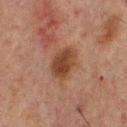Q: Was this lesion biopsied?
A: total-body-photography surveillance lesion; no biopsy
Q: Lesion location?
A: the upper back
Q: How was this image acquired?
A: total-body-photography crop, ~15 mm field of view
Q: Automated lesion metrics?
A: a footprint of about 8 mm² and an outline eccentricity of about 0.75 (0 = round, 1 = elongated); a normalized lesion–skin contrast near 9.5; border irregularity of about 2 on a 0–10 scale and peripheral color asymmetry of about 1; a classifier nevus-likeness of about 95/100
Q: Illumination type?
A: cross-polarized
Q: Who is the patient?
A: male, approximately 65 years of age
Q: How large is the lesion?
A: ~4 mm (longest diameter)